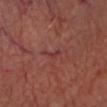Captured during whole-body skin photography for melanoma surveillance; the lesion was not biopsied.
The lesion-visualizer software estimated a lesion area of about 2 mm², a shape eccentricity near 0.85, and two-axis asymmetry of about 0.65. And it measured a lesion color around L≈35 a*≈26 b*≈20 in CIELAB, a lesion–skin lightness drop of about 6, and a normalized lesion–skin contrast near 5.5. The software also gave a border-irregularity index near 7/10.
A male patient aged 58 to 62.
This image is a 15 mm lesion crop taken from a total-body photograph.
Measured at roughly 2.5 mm in maximum diameter.
Located on the head or neck.